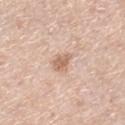Clinical impression:
Part of a total-body skin-imaging series; this lesion was reviewed on a skin check and was not flagged for biopsy.
Clinical summary:
Automated tile analysis of the lesion measured a lesion area of about 4 mm² and an eccentricity of roughly 0.8. It also reported a mean CIELAB color near L≈64 a*≈19 b*≈30, a lesion–skin lightness drop of about 11, and a lesion-to-skin contrast of about 7 (normalized; higher = more distinct). The patient is a female approximately 65 years of age. Located on the right lower leg. A close-up tile cropped from a whole-body skin photograph, about 15 mm across. Longest diameter approximately 3 mm.